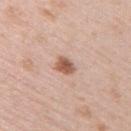Impression: Part of a total-body skin-imaging series; this lesion was reviewed on a skin check and was not flagged for biopsy. Acquisition and patient details: A female subject, aged approximately 65. This is a white-light tile. A roughly 15 mm field-of-view crop from a total-body skin photograph. About 2.5 mm across. Located on the upper back.